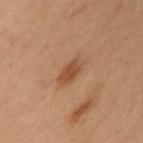{
  "biopsy_status": "not biopsied; imaged during a skin examination",
  "patient": {
    "sex": "female",
    "age_approx": 55
  },
  "site": "left upper arm",
  "image": {
    "source": "total-body photography crop",
    "field_of_view_mm": 15
  }
}